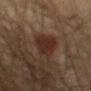| key | value |
|---|---|
| workup | no biopsy performed (imaged during a skin exam) |
| acquisition | ~15 mm crop, total-body skin-cancer survey |
| patient | male, aged 38 to 42 |
| location | the right upper arm |